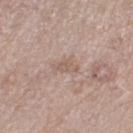Clinical summary:
A female patient, in their 60s. From the leg. The lesion's longest dimension is about 3 mm. A 15 mm close-up tile from a total-body photography series done for melanoma screening.